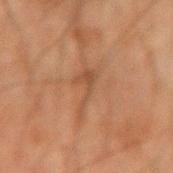  biopsy_status: not biopsied; imaged during a skin examination
  automated_metrics:
    cielab_L: 39
    cielab_a: 18
    cielab_b: 28
    vs_skin_contrast_norm: 5.5
    nevus_likeness_0_100: 0
    lesion_detection_confidence_0_100: 95
  image:
    source: total-body photography crop
    field_of_view_mm: 15
  site: right forearm
  lesion_size:
    long_diameter_mm_approx: 4.5
  patient:
    sex: male
    age_approx: 65